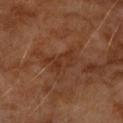Part of a total-body skin-imaging series; this lesion was reviewed on a skin check and was not flagged for biopsy.
From the left upper arm.
The subject is a male aged 58–62.
A close-up tile cropped from a whole-body skin photograph, about 15 mm across.